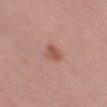workup: imaged on a skin check; not biopsied | image-analysis metrics: a footprint of about 4.5 mm², a shape eccentricity near 0.75, and a shape-asymmetry score of about 0.2 (0 = symmetric); a mean CIELAB color near L≈54 a*≈23 b*≈28, a lesion–skin lightness drop of about 9, and a normalized border contrast of about 7; an automated nevus-likeness rating near 95 out of 100 | location: the left thigh | image source: total-body-photography crop, ~15 mm field of view | lesion diameter: ≈3 mm | subject: female, aged 38–42.